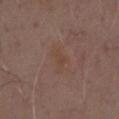<record>
  <biopsy_status>not biopsied; imaged during a skin examination</biopsy_status>
  <image>
    <source>total-body photography crop</source>
    <field_of_view_mm>15</field_of_view_mm>
  </image>
  <lighting>white-light</lighting>
  <patient>
    <sex>male</sex>
    <age_approx>70</age_approx>
  </patient>
  <lesion_size>
    <long_diameter_mm_approx>3.0</long_diameter_mm_approx>
  </lesion_size>
  <automated_metrics>
    <cielab_L>43</cielab_L>
    <cielab_a>17</cielab_a>
    <cielab_b>25</cielab_b>
    <vs_skin_contrast_norm>5.0</vs_skin_contrast_norm>
    <peripheral_color_asymmetry>0.5</peripheral_color_asymmetry>
    <nevus_likeness_0_100>0</nevus_likeness_0_100>
    <lesion_detection_confidence_0_100>100</lesion_detection_confidence_0_100>
  </automated_metrics>
  <site>chest</site>
</record>